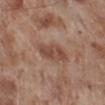Imaged during a routine full-body skin examination; the lesion was not biopsied and no histopathology is available.
This is a white-light tile.
A 15 mm crop from a total-body photograph taken for skin-cancer surveillance.
The recorded lesion diameter is about 5 mm.
A male patient, aged 68 to 72.
The lesion is on the right lower leg.